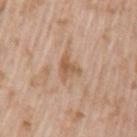Recorded during total-body skin imaging; not selected for excision or biopsy. The lesion is located on the left upper arm. The tile uses white-light illumination. Automated image analysis of the tile measured an eccentricity of roughly 0.6 and a shape-asymmetry score of about 0.5 (0 = symmetric). And it measured a border-irregularity index near 5/10, internal color variation of about 3.5 on a 0–10 scale, and peripheral color asymmetry of about 1. Measured at roughly 3 mm in maximum diameter. A male subject about 60 years old. Cropped from a whole-body photographic skin survey; the tile spans about 15 mm.